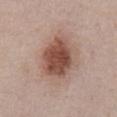* biopsy status · imaged on a skin check; not biopsied
* imaging modality · 15 mm crop, total-body photography
* patient · male, aged around 55
* lesion diameter · about 5.5 mm
* anatomic site · the abdomen
* tile lighting · white-light illumination
* automated metrics · a border-irregularity rating of about 1.5/10, a within-lesion color-variation index near 5.5/10, and radial color variation of about 1.5; a classifier nevus-likeness of about 95/100 and lesion-presence confidence of about 100/100